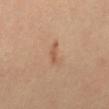Clinical impression: The lesion was photographed on a routine skin check and not biopsied; there is no pathology result. Clinical summary: This is a cross-polarized tile. On the abdomen. A male patient in their 40s. Approximately 2.5 mm at its widest. Automated tile analysis of the lesion measured a shape eccentricity near 0.95 and two-axis asymmetry of about 0.35. It also reported a border-irregularity rating of about 4/10 and radial color variation of about 0. Cropped from a whole-body photographic skin survey; the tile spans about 15 mm.